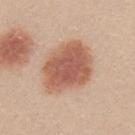{
  "biopsy_status": "not biopsied; imaged during a skin examination"
}